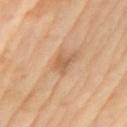acquisition = ~15 mm crop, total-body skin-cancer survey | location = the arm | patient = female, about 70 years old.